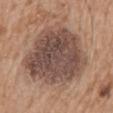follow-up — imaged on a skin check; not biopsied | location — the mid back | lighting — white-light illumination | size — ≈9 mm | automated metrics — a footprint of about 49 mm², a shape eccentricity near 0.55, and a shape-asymmetry score of about 0.2 (0 = symmetric); a normalized border contrast of about 10 | subject — male, aged 68–72 | acquisition — ~15 mm crop, total-body skin-cancer survey.